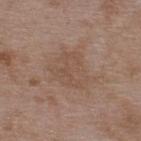  patient:
    sex: male
    age_approx: 50
  site: back
  image:
    source: total-body photography crop
    field_of_view_mm: 15